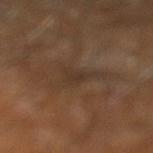A male patient, in their 60s.
The total-body-photography lesion software estimated a lesion area of about 4.5 mm², an outline eccentricity of about 0.6 (0 = round, 1 = elongated), and a shape-asymmetry score of about 0.5 (0 = symmetric). The analysis additionally found about 4 CIELAB-L* units darker than the surrounding skin and a normalized lesion–skin contrast near 4.5. The analysis additionally found a color-variation rating of about 1.5/10 and peripheral color asymmetry of about 0.5. The software also gave an automated nevus-likeness rating near 0 out of 100 and a detector confidence of about 60 out of 100 that the crop contains a lesion.
On the leg.
Measured at roughly 3 mm in maximum diameter.
This image is a 15 mm lesion crop taken from a total-body photograph.
The tile uses cross-polarized illumination.